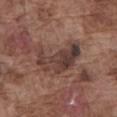Clinical impression: Imaged during a routine full-body skin examination; the lesion was not biopsied and no histopathology is available. Context: About 6 mm across. Automated image analysis of the tile measured a footprint of about 17 mm² and an outline eccentricity of about 0.8 (0 = round, 1 = elongated). It also reported a nevus-likeness score of about 0/100. Captured under white-light illumination. A male patient about 75 years old. The lesion is located on the abdomen. A roughly 15 mm field-of-view crop from a total-body skin photograph.